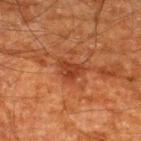| field | value |
|---|---|
| biopsy status | total-body-photography surveillance lesion; no biopsy |
| body site | the upper back |
| image-analysis metrics | a lesion area of about 5.5 mm², an eccentricity of roughly 0.65, and a shape-asymmetry score of about 0.45 (0 = symmetric); an average lesion color of about L≈32 a*≈24 b*≈30 (CIELAB), a lesion–skin lightness drop of about 7, and a normalized lesion–skin contrast near 7; a border-irregularity index near 4/10, a within-lesion color-variation index near 2.5/10, and peripheral color asymmetry of about 1; a detector confidence of about 100 out of 100 that the crop contains a lesion |
| image source | total-body-photography crop, ~15 mm field of view |
| subject | male, approximately 60 years of age |
| tile lighting | cross-polarized |